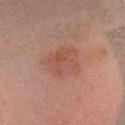Findings:
• workup — catalogued during a skin exam; not biopsied
• subject — male, aged approximately 30
• acquisition — ~15 mm crop, total-body skin-cancer survey
• body site — the head or neck